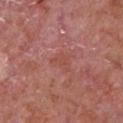Clinical summary:
The patient is a male in their mid-60s. Approximately 3 mm at its widest. Cropped from a total-body skin-imaging series; the visible field is about 15 mm. From the chest. An algorithmic analysis of the crop reported a normalized border contrast of about 5. The analysis additionally found a border-irregularity index near 5.5/10, internal color variation of about 1 on a 0–10 scale, and radial color variation of about 0.5. It also reported a classifier nevus-likeness of about 0/100 and a detector confidence of about 100 out of 100 that the crop contains a lesion. The tile uses white-light illumination.